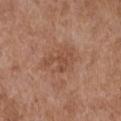| field | value |
|---|---|
| workup | no biopsy performed (imaged during a skin exam) |
| lighting | white-light illumination |
| image-analysis metrics | a shape eccentricity near 0.7 and two-axis asymmetry of about 0.25 |
| image | total-body-photography crop, ~15 mm field of view |
| patient | female, aged approximately 75 |
| site | the front of the torso |